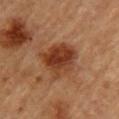The lesion was tiled from a total-body skin photograph and was not biopsied. A female patient aged around 60. Imaged with cross-polarized lighting. A 15 mm crop from a total-body photograph taken for skin-cancer surveillance. An algorithmic analysis of the crop reported an area of roughly 16 mm², an outline eccentricity of about 0.35 (0 = round, 1 = elongated), and two-axis asymmetry of about 0.25. Located on the back. About 4.5 mm across.